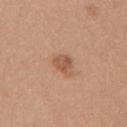Impression: The lesion was photographed on a routine skin check and not biopsied; there is no pathology result. Clinical summary: From the mid back. A 15 mm crop from a total-body photograph taken for skin-cancer surveillance. A female subject, in their mid- to late 30s. Approximately 3 mm at its widest. This is a white-light tile.